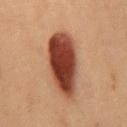The subject is a female aged 38 to 42.
The lesion-visualizer software estimated a lesion area of about 22 mm² and an outline eccentricity of about 0.8 (0 = round, 1 = elongated). The analysis additionally found a border-irregularity rating of about 2/10, a within-lesion color-variation index near 6/10, and peripheral color asymmetry of about 2. The software also gave a classifier nevus-likeness of about 100/100 and lesion-presence confidence of about 100/100.
The lesion's longest dimension is about 7 mm.
Captured under cross-polarized illumination.
On the mid back.
A 15 mm close-up tile from a total-body photography series done for melanoma screening.